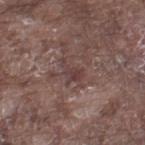Impression: This lesion was catalogued during total-body skin photography and was not selected for biopsy. Clinical summary: A male patient, aged 73 to 77. Cropped from a whole-body photographic skin survey; the tile spans about 15 mm. This is a white-light tile. The lesion-visualizer software estimated roughly 7 lightness units darker than nearby skin and a lesion-to-skin contrast of about 6.5 (normalized; higher = more distinct). And it measured border irregularity of about 6 on a 0–10 scale, internal color variation of about 1.5 on a 0–10 scale, and peripheral color asymmetry of about 0.5. The software also gave a nevus-likeness score of about 0/100 and a lesion-detection confidence of about 50/100. The lesion is on the left thigh.